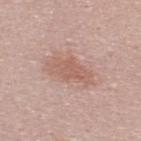No biopsy was performed on this lesion — it was imaged during a full skin examination and was not determined to be concerning. On the upper back. Longest diameter approximately 5 mm. The patient is a male aged approximately 25. A 15 mm close-up tile from a total-body photography series done for melanoma screening.